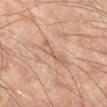Notes:
– biopsy status — no biopsy performed (imaged during a skin exam)
– site — the right lower leg
– size — about 4 mm
– image-analysis metrics — an area of roughly 5 mm², an eccentricity of roughly 0.95, and two-axis asymmetry of about 0.35; a lesion–skin lightness drop of about 7 and a lesion-to-skin contrast of about 5 (normalized; higher = more distinct)
– subject — male, aged approximately 55
– imaging modality — ~15 mm tile from a whole-body skin photo
– illumination — cross-polarized